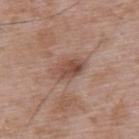About 3.5 mm across. On the upper back. A male patient, aged approximately 50. The total-body-photography lesion software estimated an area of roughly 6 mm², an outline eccentricity of about 0.8 (0 = round, 1 = elongated), and a symmetry-axis asymmetry near 0.15. The analysis additionally found a normalized lesion–skin contrast near 7.5. And it measured a border-irregularity rating of about 2/10 and a within-lesion color-variation index near 4.5/10. And it measured an automated nevus-likeness rating near 0 out of 100. This image is a 15 mm lesion crop taken from a total-body photograph.